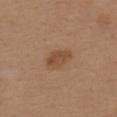Recorded during total-body skin imaging; not selected for excision or biopsy.
A 15 mm crop from a total-body photograph taken for skin-cancer surveillance.
About 3.5 mm across.
The patient is a female approximately 40 years of age.
Located on the upper back.